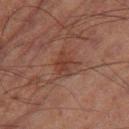Part of a total-body skin-imaging series; this lesion was reviewed on a skin check and was not flagged for biopsy.
The lesion is located on the right thigh.
The patient is a male about 70 years old.
The lesion's longest dimension is about 3 mm.
A roughly 15 mm field-of-view crop from a total-body skin photograph.
Imaged with cross-polarized lighting.
The total-body-photography lesion software estimated a lesion area of about 5.5 mm² and a shape eccentricity near 0.65. The analysis additionally found a normalized lesion–skin contrast near 6. And it measured a border-irregularity rating of about 3.5/10, a within-lesion color-variation index near 2.5/10, and radial color variation of about 1. The analysis additionally found a detector confidence of about 100 out of 100 that the crop contains a lesion.